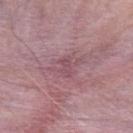follow-up=total-body-photography surveillance lesion; no biopsy
subject=male, approximately 65 years of age
size=≈2.5 mm
automated lesion analysis=a mean CIELAB color near L≈49 a*≈25 b*≈14 and a normalized border contrast of about 5; a classifier nevus-likeness of about 0/100 and a lesion-detection confidence of about 55/100
imaging modality=~15 mm crop, total-body skin-cancer survey
site=the left upper arm